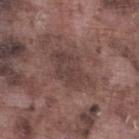Impression:
Part of a total-body skin-imaging series; this lesion was reviewed on a skin check and was not flagged for biopsy.
Acquisition and patient details:
A male patient aged around 75. Cropped from a whole-body photographic skin survey; the tile spans about 15 mm. Longest diameter approximately 5 mm. Located on the left lower leg. This is a white-light tile.